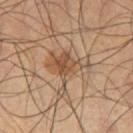biopsy status: no biopsy performed (imaged during a skin exam) | subject: male, aged 58 to 62 | illumination: cross-polarized illumination | lesion size: about 5.5 mm | body site: the left thigh | automated lesion analysis: a lesion–skin lightness drop of about 9 and a normalized border contrast of about 6.5; border irregularity of about 7.5 on a 0–10 scale, internal color variation of about 5.5 on a 0–10 scale, and peripheral color asymmetry of about 2; an automated nevus-likeness rating near 60 out of 100 and lesion-presence confidence of about 100/100 | imaging modality: ~15 mm tile from a whole-body skin photo.